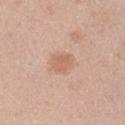Q: Was this lesion biopsied?
A: imaged on a skin check; not biopsied
Q: How was this image acquired?
A: total-body-photography crop, ~15 mm field of view
Q: How large is the lesion?
A: ≈2.5 mm
Q: What is the anatomic site?
A: the arm
Q: How was the tile lit?
A: white-light
Q: Automated lesion metrics?
A: an average lesion color of about L≈62 a*≈22 b*≈32 (CIELAB), a lesion–skin lightness drop of about 8, and a normalized lesion–skin contrast near 6; lesion-presence confidence of about 100/100
Q: Patient demographics?
A: male, approximately 45 years of age